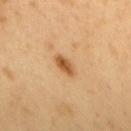Findings:
– size — about 3.5 mm
– tile lighting — cross-polarized illumination
– site — the upper back
– acquisition — ~15 mm crop, total-body skin-cancer survey
– subject — male, aged around 50